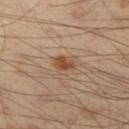Background:
A close-up tile cropped from a whole-body skin photograph, about 15 mm across. The lesion is located on the left thigh. An algorithmic analysis of the crop reported a lesion area of about 4.5 mm² and an eccentricity of roughly 0.7. The analysis additionally found an average lesion color of about L≈49 a*≈19 b*≈32 (CIELAB) and a normalized border contrast of about 8.5. About 2.5 mm across. A male subject about 40 years old.